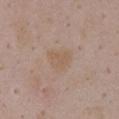<lesion>
  <biopsy_status>not biopsied; imaged during a skin examination</biopsy_status>
  <image>
    <source>total-body photography crop</source>
    <field_of_view_mm>15</field_of_view_mm>
  </image>
  <site>chest</site>
  <patient>
    <sex>female</sex>
    <age_approx>35</age_approx>
  </patient>
</lesion>